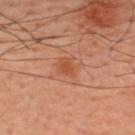The lesion was tiled from a total-body skin photograph and was not biopsied.
Approximately 2.5 mm at its widest.
A lesion tile, about 15 mm wide, cut from a 3D total-body photograph.
A male subject aged 58 to 62.
Imaged with cross-polarized lighting.
The lesion is on the back.